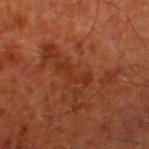– lighting · cross-polarized illumination
– size · ~4 mm (longest diameter)
– patient · male, about 80 years old
– acquisition · ~15 mm tile from a whole-body skin photo
– location · the right lower leg